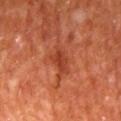Captured during whole-body skin photography for melanoma surveillance; the lesion was not biopsied.
Automated tile analysis of the lesion measured an area of roughly 5.5 mm² and a shape eccentricity near 0.8. And it measured internal color variation of about 2.5 on a 0–10 scale and a peripheral color-asymmetry measure near 1.
On the mid back.
Captured under cross-polarized illumination.
A male subject, aged 63–67.
A lesion tile, about 15 mm wide, cut from a 3D total-body photograph.